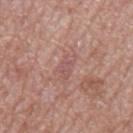notes — imaged on a skin check; not biopsied | diameter — about 3 mm | automated metrics — a symmetry-axis asymmetry near 0.35; an average lesion color of about L≈54 a*≈22 b*≈22 (CIELAB), a lesion–skin lightness drop of about 6, and a normalized lesion–skin contrast near 5; a nevus-likeness score of about 0/100 and a lesion-detection confidence of about 70/100 | subject — male, approximately 65 years of age | site — the mid back | illumination — white-light illumination | image source — ~15 mm tile from a whole-body skin photo.